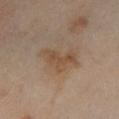Impression: This lesion was catalogued during total-body skin photography and was not selected for biopsy. Clinical summary: A close-up tile cropped from a whole-body skin photograph, about 15 mm across. About 5 mm across. A male subject, about 65 years old. From the left lower leg.